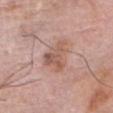Assessment: Imaged during a routine full-body skin examination; the lesion was not biopsied and no histopathology is available. Clinical summary: The lesion is located on the head or neck. A male patient, roughly 80 years of age. The lesion-visualizer software estimated an average lesion color of about L≈57 a*≈21 b*≈27 (CIELAB), about 9 CIELAB-L* units darker than the surrounding skin, and a normalized border contrast of about 6.5. It also reported radial color variation of about 1.5. The tile uses white-light illumination. Cropped from a whole-body photographic skin survey; the tile spans about 15 mm.